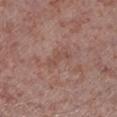Captured during whole-body skin photography for melanoma surveillance; the lesion was not biopsied. A region of skin cropped from a whole-body photographic capture, roughly 15 mm wide. The lesion is on the left lower leg. A male subject aged 53 to 57. Captured under white-light illumination.